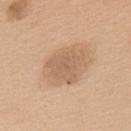No biopsy was performed on this lesion — it was imaged during a full skin examination and was not determined to be concerning.
A region of skin cropped from a whole-body photographic capture, roughly 15 mm wide.
Located on the upper back.
The total-body-photography lesion software estimated a lesion color around L≈61 a*≈18 b*≈33 in CIELAB, a lesion–skin lightness drop of about 9, and a normalized border contrast of about 5.5. It also reported border irregularity of about 4.5 on a 0–10 scale.
The patient is a male aged around 60.
About 5 mm across.
Captured under white-light illumination.